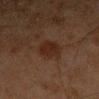Q: Is there a histopathology result?
A: no biopsy performed (imaged during a skin exam)
Q: Who is the patient?
A: male, aged 43 to 47
Q: Illumination type?
A: cross-polarized
Q: What is the lesion's diameter?
A: ≈3.5 mm
Q: What is the imaging modality?
A: total-body-photography crop, ~15 mm field of view
Q: Lesion location?
A: the left forearm